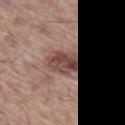The lesion was photographed on a routine skin check and not biopsied; there is no pathology result. Approximately 3.5 mm at its widest. The lesion-visualizer software estimated a footprint of about 10 mm², a shape eccentricity near 0.5, and two-axis asymmetry of about 0.2. The software also gave a lesion–skin lightness drop of about 13 and a lesion-to-skin contrast of about 9.5 (normalized; higher = more distinct). The software also gave an automated nevus-likeness rating near 0 out of 100 and lesion-presence confidence of about 95/100. The lesion is on the left thigh. The subject is a male aged 63–67. The tile uses white-light illumination. A close-up tile cropped from a whole-body skin photograph, about 15 mm across.